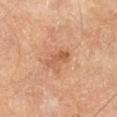<case>
  <biopsy_status>not biopsied; imaged during a skin examination</biopsy_status>
  <patient>
    <sex>male</sex>
    <age_approx>65</age_approx>
  </patient>
  <site>leg</site>
  <lesion_size>
    <long_diameter_mm_approx>3.5</long_diameter_mm_approx>
  </lesion_size>
  <image>
    <source>total-body photography crop</source>
    <field_of_view_mm>15</field_of_view_mm>
  </image>
</case>